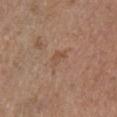Notes:
• follow-up — no biopsy performed (imaged during a skin exam)
• patient — female, in their mid-60s
• automated metrics — a lesion color around L≈51 a*≈18 b*≈30 in CIELAB; a border-irregularity index near 3.5/10 and a within-lesion color-variation index near 2/10; an automated nevus-likeness rating near 0 out of 100
• lighting — white-light illumination
• diameter — about 3 mm
• site — the right upper arm
• acquisition — ~15 mm crop, total-body skin-cancer survey